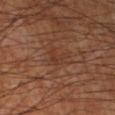Assessment: No biopsy was performed on this lesion — it was imaged during a full skin examination and was not determined to be concerning. Context: Approximately 2.5 mm at its widest. The tile uses cross-polarized illumination. The lesion is on the right lower leg. The patient is a male aged 58 to 62. A close-up tile cropped from a whole-body skin photograph, about 15 mm across. Automated image analysis of the tile measured a footprint of about 3 mm², an outline eccentricity of about 0.75 (0 = round, 1 = elongated), and two-axis asymmetry of about 0.6. And it measured a lesion color around L≈35 a*≈21 b*≈28 in CIELAB, a lesion–skin lightness drop of about 5, and a normalized border contrast of about 5. The analysis additionally found a color-variation rating of about 0/10 and radial color variation of about 0. The software also gave a lesion-detection confidence of about 95/100.